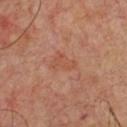Impression: This lesion was catalogued during total-body skin photography and was not selected for biopsy. Acquisition and patient details: On the chest. A 15 mm crop from a total-body photograph taken for skin-cancer surveillance. A male patient, aged 53 to 57.